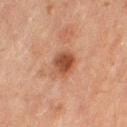{
  "biopsy_status": "not biopsied; imaged during a skin examination",
  "site": "leg",
  "lesion_size": {
    "long_diameter_mm_approx": 3.5
  },
  "patient": {
    "sex": "female",
    "age_approx": 55
  },
  "lighting": "cross-polarized",
  "automated_metrics": {
    "eccentricity": 0.6,
    "shape_asymmetry": 0.15,
    "border_irregularity_0_10": 1.5,
    "peripheral_color_asymmetry": 1.5
  },
  "image": {
    "source": "total-body photography crop",
    "field_of_view_mm": 15
  }
}